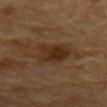  biopsy_status: not biopsied; imaged during a skin examination
  lesion_size:
    long_diameter_mm_approx: 4.0
  lighting: cross-polarized
  patient:
    sex: male
    age_approx: 85+
  site: mid back
  automated_metrics:
    cielab_L: 24
    cielab_a: 15
    cielab_b: 25
    vs_skin_darker_L: 7.0
    border_irregularity_0_10: 2.5
    color_variation_0_10: 3.5
    peripheral_color_asymmetry: 1.0
  image:
    source: total-body photography crop
    field_of_view_mm: 15